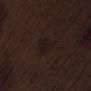{
  "biopsy_status": "not biopsied; imaged during a skin examination",
  "patient": {
    "sex": "male",
    "age_approx": 70
  },
  "lesion_size": {
    "long_diameter_mm_approx": 3.0
  },
  "image": {
    "source": "total-body photography crop",
    "field_of_view_mm": 15
  },
  "site": "right thigh",
  "lighting": "white-light"
}